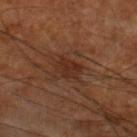Notes:
• image source — ~15 mm tile from a whole-body skin photo
• automated lesion analysis — a lesion area of about 5.5 mm², a shape eccentricity near 0.75, and two-axis asymmetry of about 0.2; about 6 CIELAB-L* units darker than the surrounding skin and a normalized lesion–skin contrast near 6.5; a border-irregularity rating of about 2.5/10, a within-lesion color-variation index near 3/10, and peripheral color asymmetry of about 1; a nevus-likeness score of about 0/100 and lesion-presence confidence of about 100/100
• tile lighting — cross-polarized
• subject — male, about 60 years old
• anatomic site — the leg
• lesion size — ≈3 mm